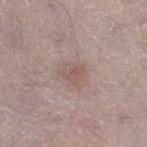Part of a total-body skin-imaging series; this lesion was reviewed on a skin check and was not flagged for biopsy.
An algorithmic analysis of the crop reported a lesion area of about 4.5 mm² and an eccentricity of roughly 0.55. And it measured border irregularity of about 2.5 on a 0–10 scale.
The lesion is located on the right thigh.
A lesion tile, about 15 mm wide, cut from a 3D total-body photograph.
The subject is a male about 75 years old.
About 2.5 mm across.